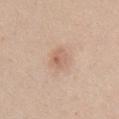Captured during whole-body skin photography for melanoma surveillance; the lesion was not biopsied. The subject is a female aged around 40. Automated image analysis of the tile measured an area of roughly 4.5 mm² and an outline eccentricity of about 0.7 (0 = round, 1 = elongated). The software also gave a within-lesion color-variation index near 4/10 and a peripheral color-asymmetry measure near 1.5. The software also gave a classifier nevus-likeness of about 30/100 and a detector confidence of about 100 out of 100 that the crop contains a lesion. Measured at roughly 3 mm in maximum diameter. The lesion is on the chest. Imaged with white-light lighting. A region of skin cropped from a whole-body photographic capture, roughly 15 mm wide.